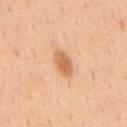The lesion was tiled from a total-body skin photograph and was not biopsied.
A lesion tile, about 15 mm wide, cut from a 3D total-body photograph.
An algorithmic analysis of the crop reported about 12 CIELAB-L* units darker than the surrounding skin and a normalized lesion–skin contrast near 7.5. The analysis additionally found a border-irregularity index near 2.5/10, a color-variation rating of about 2/10, and radial color variation of about 0.5. And it measured a classifier nevus-likeness of about 90/100 and lesion-presence confidence of about 100/100.
The lesion is located on the chest.
The patient is a male aged 48–52.
The tile uses white-light illumination.
Longest diameter approximately 3.5 mm.